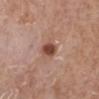Clinical impression:
This lesion was catalogued during total-body skin photography and was not selected for biopsy.
Clinical summary:
This image is a 15 mm lesion crop taken from a total-body photograph. A female patient aged 73 to 77. Imaged with white-light lighting. The lesion's longest dimension is about 2.5 mm. The lesion-visualizer software estimated a lesion area of about 4.5 mm² and a shape-asymmetry score of about 0.2 (0 = symmetric). And it measured an average lesion color of about L≈47 a*≈23 b*≈27 (CIELAB), roughly 13 lightness units darker than nearby skin, and a normalized border contrast of about 9.5. And it measured a border-irregularity index near 1.5/10, a within-lesion color-variation index near 2.5/10, and peripheral color asymmetry of about 1. And it measured a classifier nevus-likeness of about 95/100 and lesion-presence confidence of about 100/100. The lesion is located on the left lower leg.